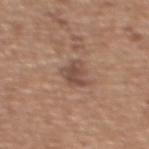Q: How was this image acquired?
A: ~15 mm crop, total-body skin-cancer survey
Q: What is the lesion's diameter?
A: ~2.5 mm (longest diameter)
Q: Where on the body is the lesion?
A: the upper back
Q: Who is the patient?
A: female, in their 60s
Q: How was the tile lit?
A: white-light illumination